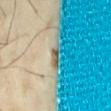notes=total-body-photography surveillance lesion; no biopsy | image-analysis metrics=an eccentricity of roughly 0.85; a border-irregularity index near 2.5/10, internal color variation of about 0 on a 0–10 scale, and a peripheral color-asymmetry measure near 0 | size=~2 mm (longest diameter) | image=~15 mm crop, total-body skin-cancer survey | subject=male, about 40 years old | body site=the lower back | tile lighting=cross-polarized.